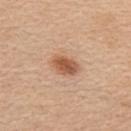This lesion was catalogued during total-body skin photography and was not selected for biopsy.
The lesion is on the upper back.
A male patient, aged approximately 60.
Imaged with white-light lighting.
Automated tile analysis of the lesion measured a shape eccentricity near 0.75 and two-axis asymmetry of about 0.2. The software also gave a border-irregularity index near 1.5/10, internal color variation of about 3.5 on a 0–10 scale, and a peripheral color-asymmetry measure near 1.
Measured at roughly 3.5 mm in maximum diameter.
This image is a 15 mm lesion crop taken from a total-body photograph.